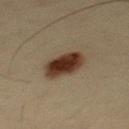• follow-up · catalogued during a skin exam; not biopsied
• location · the mid back
• acquisition · ~15 mm crop, total-body skin-cancer survey
• subject · male, aged approximately 35